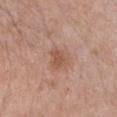Q: How was the tile lit?
A: white-light
Q: How large is the lesion?
A: ~2.5 mm (longest diameter)
Q: How was this image acquired?
A: ~15 mm crop, total-body skin-cancer survey
Q: Lesion location?
A: the chest
Q: Who is the patient?
A: female, roughly 60 years of age